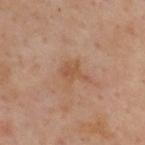| field | value |
|---|---|
| follow-up | total-body-photography surveillance lesion; no biopsy |
| image | total-body-photography crop, ~15 mm field of view |
| patient | male, aged 53 to 57 |
| body site | the back |